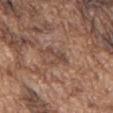Part of a total-body skin-imaging series; this lesion was reviewed on a skin check and was not flagged for biopsy. The patient is a male in their mid-70s. This is a white-light tile. From the chest. A region of skin cropped from a whole-body photographic capture, roughly 15 mm wide. Automated image analysis of the tile measured an area of roughly 4.5 mm² and a symmetry-axis asymmetry near 0.5. The software also gave border irregularity of about 6.5 on a 0–10 scale and radial color variation of about 0.5.